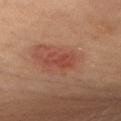Findings:
* workup: imaged on a skin check; not biopsied
* site: the upper back
* lesion size: ≈4 mm
* subject: female, aged around 50
* imaging modality: total-body-photography crop, ~15 mm field of view
* tile lighting: cross-polarized illumination